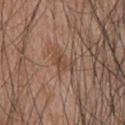{
  "site": "back",
  "image": {
    "source": "total-body photography crop",
    "field_of_view_mm": 15
  },
  "lesion_size": {
    "long_diameter_mm_approx": 3.0
  },
  "patient": {
    "sex": "male",
    "age_approx": 45
  },
  "automated_metrics": {
    "shape_asymmetry": 0.65,
    "cielab_L": 45,
    "cielab_a": 18,
    "cielab_b": 27,
    "vs_skin_darker_L": 8.0,
    "vs_skin_contrast_norm": 6.0,
    "nevus_likeness_0_100": 0,
    "lesion_detection_confidence_0_100": 100
  },
  "lighting": "white-light"
}